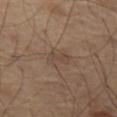Cropped from a whole-body photographic skin survey; the tile spans about 15 mm. On the left thigh. Longest diameter approximately 3 mm. An algorithmic analysis of the crop reported an average lesion color of about L≈45 a*≈15 b*≈25 (CIELAB), about 6 CIELAB-L* units darker than the surrounding skin, and a normalized border contrast of about 5. Imaged with cross-polarized lighting.